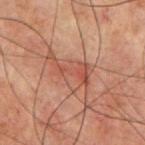  biopsy_status: not biopsied; imaged during a skin examination
  site: chest
  image:
    source: total-body photography crop
    field_of_view_mm: 15
  patient:
    sex: male
    age_approx: 70
  automated_metrics:
    cielab_L: 39
    cielab_a: 20
    cielab_b: 24
    vs_skin_darker_L: 6.0
    border_irregularity_0_10: 5.5
    nevus_likeness_0_100: 5
    lesion_detection_confidence_0_100: 95